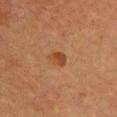Assessment: Part of a total-body skin-imaging series; this lesion was reviewed on a skin check and was not flagged for biopsy.